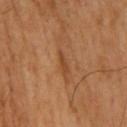imaging modality: ~15 mm tile from a whole-body skin photo
lesion diameter: ~3 mm (longest diameter)
site: the head or neck
subject: male, aged around 70